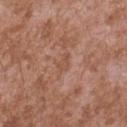Part of a total-body skin-imaging series; this lesion was reviewed on a skin check and was not flagged for biopsy.
The lesion-visualizer software estimated about 6 CIELAB-L* units darker than the surrounding skin and a lesion-to-skin contrast of about 4.5 (normalized; higher = more distinct). And it measured a border-irregularity index near 3.5/10 and radial color variation of about 0.5.
A male subject aged around 45.
A 15 mm crop from a total-body photograph taken for skin-cancer surveillance.
The tile uses white-light illumination.
From the back.